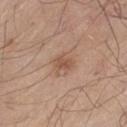Captured during whole-body skin photography for melanoma surveillance; the lesion was not biopsied.